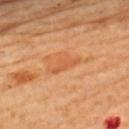automated_metrics:
  border_irregularity_0_10: 5.5
  color_variation_0_10: 1.0
  nevus_likeness_0_100: 0
  lesion_detection_confidence_0_100: 100
image:
  source: total-body photography crop
  field_of_view_mm: 15
site: upper back
lesion_size:
  long_diameter_mm_approx: 4.0
lighting: cross-polarized
patient:
  sex: female
  age_approx: 65